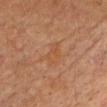follow-up: total-body-photography surveillance lesion; no biopsy
image: total-body-photography crop, ~15 mm field of view
subject: female, aged 78 to 82
anatomic site: the head or neck